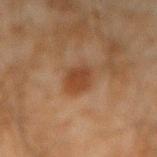diameter = ≈3.5 mm
patient = male, aged 43–47
TBP lesion metrics = an average lesion color of about L≈35 a*≈19 b*≈29 (CIELAB) and roughly 8 lightness units darker than nearby skin; border irregularity of about 2 on a 0–10 scale, internal color variation of about 2.5 on a 0–10 scale, and a peripheral color-asymmetry measure near 1
anatomic site = the arm
illumination = cross-polarized
acquisition = ~15 mm crop, total-body skin-cancer survey